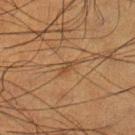Assessment:
Part of a total-body skin-imaging series; this lesion was reviewed on a skin check and was not flagged for biopsy.
Context:
A close-up tile cropped from a whole-body skin photograph, about 15 mm across. A male patient, aged approximately 50. Located on the left lower leg. Approximately 3 mm at its widest. Imaged with cross-polarized lighting.